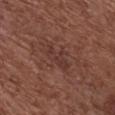Impression:
Part of a total-body skin-imaging series; this lesion was reviewed on a skin check and was not flagged for biopsy.
Acquisition and patient details:
The lesion-visualizer software estimated an area of roughly 6.5 mm², a shape eccentricity near 0.9, and a symmetry-axis asymmetry near 0.3. It also reported a border-irregularity rating of about 4/10, internal color variation of about 2 on a 0–10 scale, and peripheral color asymmetry of about 0.5. The lesion is on the front of the torso. The tile uses white-light illumination. Approximately 4 mm at its widest. A female subject approximately 80 years of age. Cropped from a total-body skin-imaging series; the visible field is about 15 mm.